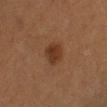biopsy status: total-body-photography surveillance lesion; no biopsy | site: the right lower leg | automated metrics: a lesion area of about 6 mm², a shape eccentricity near 0.45, and a shape-asymmetry score of about 0.15 (0 = symmetric); roughly 7 lightness units darker than nearby skin; a within-lesion color-variation index near 2/10 and peripheral color asymmetry of about 0.5 | acquisition: ~15 mm tile from a whole-body skin photo | patient: female, aged 53–57 | size: ≈3 mm.